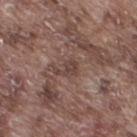<lesion>
  <biopsy_status>not biopsied; imaged during a skin examination</biopsy_status>
</lesion>